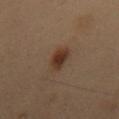Q: Was this lesion biopsied?
A: imaged on a skin check; not biopsied
Q: What lighting was used for the tile?
A: cross-polarized
Q: What kind of image is this?
A: ~15 mm tile from a whole-body skin photo
Q: Lesion location?
A: the mid back
Q: What is the lesion's diameter?
A: ≈3 mm
Q: What are the patient's age and sex?
A: male, aged 53–57